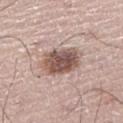Cropped from a whole-body photographic skin survey; the tile spans about 15 mm.
The recorded lesion diameter is about 5 mm.
Captured under white-light illumination.
A male patient, in their 70s.
The lesion is located on the right leg.